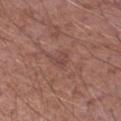workup — catalogued during a skin exam; not biopsied | location — the right upper arm | subject — male, aged approximately 70 | lesion diameter — ≈2.5 mm | image — ~15 mm tile from a whole-body skin photo.